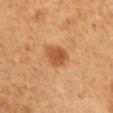<case>
<biopsy_status>not biopsied; imaged during a skin examination</biopsy_status>
<patient>
  <sex>male</sex>
  <age_approx>50</age_approx>
</patient>
<lesion_size>
  <long_diameter_mm_approx>3.0</long_diameter_mm_approx>
</lesion_size>
<image>
  <source>total-body photography crop</source>
  <field_of_view_mm>15</field_of_view_mm>
</image>
<site>left upper arm</site>
<lighting>cross-polarized</lighting>
</case>